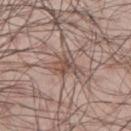Recorded during total-body skin imaging; not selected for excision or biopsy. Captured under white-light illumination. Located on the right upper arm. The recorded lesion diameter is about 4 mm. A 15 mm close-up extracted from a 3D total-body photography capture. A male patient, aged around 45.